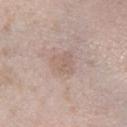The lesion was tiled from a total-body skin photograph and was not biopsied.
Automated tile analysis of the lesion measured an area of roughly 6 mm², an eccentricity of roughly 0.5, and a symmetry-axis asymmetry near 0.3. It also reported a mean CIELAB color near L≈60 a*≈15 b*≈24 and a lesion–skin lightness drop of about 6.
A close-up tile cropped from a whole-body skin photograph, about 15 mm across.
A female patient aged 53–57.
Approximately 3 mm at its widest.
The lesion is on the left lower leg.
Captured under white-light illumination.